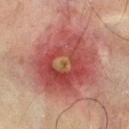Impression:
Part of a total-body skin-imaging series; this lesion was reviewed on a skin check and was not flagged for biopsy.
Image and clinical context:
On the chest. This image is a 15 mm lesion crop taken from a total-body photograph. A male patient approximately 70 years of age.